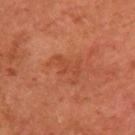biopsy status = no biopsy performed (imaged during a skin exam); body site = the right upper arm; size = ~4 mm (longest diameter); patient = female, aged 58–62; image = ~15 mm crop, total-body skin-cancer survey; lighting = cross-polarized.